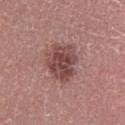biopsy_status: not biopsied; imaged during a skin examination
patient:
  sex: male
  age_approx: 20
lighting: white-light
site: left lower leg
automated_metrics:
  eccentricity: 0.6
  shape_asymmetry: 0.2
  nevus_likeness_0_100: 20
  lesion_detection_confidence_0_100: 100
image:
  source: total-body photography crop
  field_of_view_mm: 15
lesion_size:
  long_diameter_mm_approx: 5.0